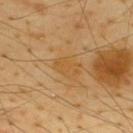biopsy status=imaged on a skin check; not biopsied
location=the back
illumination=cross-polarized illumination
lesion diameter=about 3 mm
image=total-body-photography crop, ~15 mm field of view
automated lesion analysis=a border-irregularity rating of about 4.5/10, internal color variation of about 1 on a 0–10 scale, and peripheral color asymmetry of about 0.5; an automated nevus-likeness rating near 0 out of 100
subject=male, aged around 65